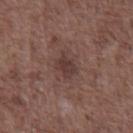Q: Is there a histopathology result?
A: total-body-photography surveillance lesion; no biopsy
Q: What is the imaging modality?
A: total-body-photography crop, ~15 mm field of view
Q: Where on the body is the lesion?
A: the abdomen
Q: Automated lesion metrics?
A: an area of roughly 4.5 mm², an outline eccentricity of about 0.6 (0 = round, 1 = elongated), and a shape-asymmetry score of about 0.25 (0 = symmetric); a lesion color around L≈36 a*≈18 b*≈20 in CIELAB and a normalized border contrast of about 7; lesion-presence confidence of about 100/100
Q: Patient demographics?
A: male, aged around 70